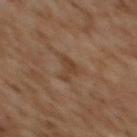follow-up = total-body-photography surveillance lesion; no biopsy | lesion size = about 3 mm | image source = ~15 mm crop, total-body skin-cancer survey | automated metrics = a mean CIELAB color near L≈35 a*≈15 b*≈26, roughly 6 lightness units darker than nearby skin, and a normalized lesion–skin contrast near 6; a border-irregularity rating of about 6/10, a color-variation rating of about 1.5/10, and radial color variation of about 0.5 | site = the upper back | subject = female, aged 58–62 | tile lighting = cross-polarized illumination.